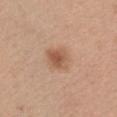Findings:
• biopsy status — imaged on a skin check; not biopsied
• image-analysis metrics — a lesion area of about 7 mm², an outline eccentricity of about 0.55 (0 = round, 1 = elongated), and a shape-asymmetry score of about 0.2 (0 = symmetric); an average lesion color of about L≈56 a*≈20 b*≈33 (CIELAB), a lesion–skin lightness drop of about 10, and a lesion-to-skin contrast of about 7.5 (normalized; higher = more distinct); a border-irregularity index near 1.5/10, internal color variation of about 3.5 on a 0–10 scale, and a peripheral color-asymmetry measure near 1
• subject — male, roughly 30 years of age
• body site — the chest
• tile lighting — white-light illumination
• imaging modality — ~15 mm tile from a whole-body skin photo